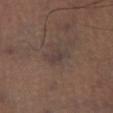This lesion was catalogued during total-body skin photography and was not selected for biopsy.
On the left leg.
The patient is a male about 50 years old.
A 15 mm close-up extracted from a 3D total-body photography capture.